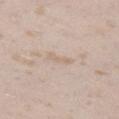Findings:
– biopsy status: no biopsy performed (imaged during a skin exam)
– imaging modality: ~15 mm crop, total-body skin-cancer survey
– lesion size: ~3 mm (longest diameter)
– patient: female, about 25 years old
– body site: the left thigh